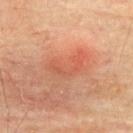Clinical impression: Part of a total-body skin-imaging series; this lesion was reviewed on a skin check and was not flagged for biopsy. Context: The subject is a male in their mid-70s. Cropped from a total-body skin-imaging series; the visible field is about 15 mm. The recorded lesion diameter is about 5 mm. Imaged with cross-polarized lighting. Automated tile analysis of the lesion measured a footprint of about 8 mm² and a shape-asymmetry score of about 0.55 (0 = symmetric). It also reported an average lesion color of about L≈48 a*≈26 b*≈30 (CIELAB), roughly 6 lightness units darker than nearby skin, and a normalized border contrast of about 4.5. On the upper back.